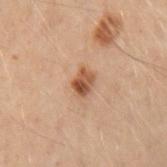Captured during whole-body skin photography for melanoma surveillance; the lesion was not biopsied.
Located on the left arm.
The recorded lesion diameter is about 2.5 mm.
Automated tile analysis of the lesion measured an area of roughly 4.5 mm², a shape eccentricity near 0.65, and a shape-asymmetry score of about 0.25 (0 = symmetric). The software also gave a within-lesion color-variation index near 6.5/10 and radial color variation of about 2.5.
Cropped from a whole-body photographic skin survey; the tile spans about 15 mm.
A female patient about 30 years old.
This is a cross-polarized tile.